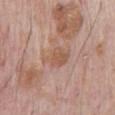Captured during whole-body skin photography for melanoma surveillance; the lesion was not biopsied.
Captured under white-light illumination.
On the chest.
A male patient, aged 68 to 72.
This image is a 15 mm lesion crop taken from a total-body photograph.
The total-body-photography lesion software estimated a lesion area of about 7.5 mm², an outline eccentricity of about 0.8 (0 = round, 1 = elongated), and two-axis asymmetry of about 0.25. It also reported a mean CIELAB color near L≈56 a*≈20 b*≈29 and a normalized border contrast of about 6.5. And it measured a classifier nevus-likeness of about 0/100 and a lesion-detection confidence of about 100/100.